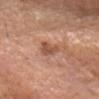Impression: Recorded during total-body skin imaging; not selected for excision or biopsy.